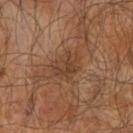Imaged during a routine full-body skin examination; the lesion was not biopsied and no histopathology is available. Measured at roughly 4 mm in maximum diameter. A lesion tile, about 15 mm wide, cut from a 3D total-body photograph. A male subject, about 65 years old. The lesion is on the right upper arm.